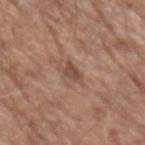<record>
<biopsy_status>not biopsied; imaged during a skin examination</biopsy_status>
<image>
  <source>total-body photography crop</source>
  <field_of_view_mm>15</field_of_view_mm>
</image>
<lesion_size>
  <long_diameter_mm_approx>2.5</long_diameter_mm_approx>
</lesion_size>
<patient>
  <sex>male</sex>
  <age_approx>70</age_approx>
</patient>
<lighting>white-light</lighting>
<site>left forearm</site>
</record>